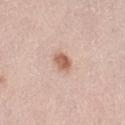Imaged during a routine full-body skin examination; the lesion was not biopsied and no histopathology is available.
A roughly 15 mm field-of-view crop from a total-body skin photograph.
On the left thigh.
The lesion's longest dimension is about 2.5 mm.
The lesion-visualizer software estimated a footprint of about 4 mm², an eccentricity of roughly 0.55, and a shape-asymmetry score of about 0.2 (0 = symmetric). The software also gave border irregularity of about 1.5 on a 0–10 scale, internal color variation of about 3 on a 0–10 scale, and a peripheral color-asymmetry measure near 1.
A female patient aged around 65.